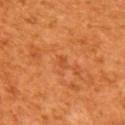Clinical impression:
No biopsy was performed on this lesion — it was imaged during a full skin examination and was not determined to be concerning.
Clinical summary:
On the back. The patient is a male aged 43–47. The total-body-photography lesion software estimated an area of roughly 1 mm². And it measured a lesion color around L≈48 a*≈30 b*≈42 in CIELAB, roughly 6 lightness units darker than nearby skin, and a lesion-to-skin contrast of about 4.5 (normalized; higher = more distinct). The software also gave a nevus-likeness score of about 0/100 and a detector confidence of about 100 out of 100 that the crop contains a lesion. A roughly 15 mm field-of-view crop from a total-body skin photograph.